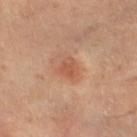{
  "biopsy_status": "not biopsied; imaged during a skin examination",
  "patient": {
    "age_approx": 60
  },
  "site": "left lower leg",
  "image": {
    "source": "total-body photography crop",
    "field_of_view_mm": 15
  }
}